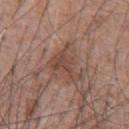A lesion tile, about 15 mm wide, cut from a 3D total-body photograph.
The recorded lesion diameter is about 4.5 mm.
The total-body-photography lesion software estimated a lesion area of about 9 mm² and a shape-asymmetry score of about 0.55 (0 = symmetric). And it measured a mean CIELAB color near L≈46 a*≈18 b*≈26, about 8 CIELAB-L* units darker than the surrounding skin, and a normalized border contrast of about 6.5. The software also gave a border-irregularity index near 7.5/10, a within-lesion color-variation index near 2.5/10, and a peripheral color-asymmetry measure near 1. The software also gave a classifier nevus-likeness of about 0/100.
The patient is a male approximately 60 years of age.
Imaged with white-light lighting.
On the chest.